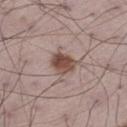Captured during whole-body skin photography for melanoma surveillance; the lesion was not biopsied.
A lesion tile, about 15 mm wide, cut from a 3D total-body photograph.
A male patient, aged around 70.
The lesion is on the left thigh.
Captured under white-light illumination.